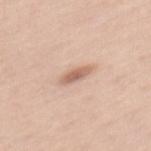Impression: The lesion was tiled from a total-body skin photograph and was not biopsied. Clinical summary: Cropped from a total-body skin-imaging series; the visible field is about 15 mm. From the mid back. Approximately 3 mm at its widest. A female subject, roughly 35 years of age. The tile uses white-light illumination.